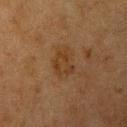Captured during whole-body skin photography for melanoma surveillance; the lesion was not biopsied. The subject is a female aged 53–57. The recorded lesion diameter is about 3 mm. The lesion-visualizer software estimated a lesion area of about 6.5 mm², a shape eccentricity near 0.55, and a shape-asymmetry score of about 0.3 (0 = symmetric). It also reported an average lesion color of about L≈31 a*≈16 b*≈28 (CIELAB). The software also gave a border-irregularity index near 3/10, a color-variation rating of about 2/10, and peripheral color asymmetry of about 0.5. On the arm. Cropped from a total-body skin-imaging series; the visible field is about 15 mm.